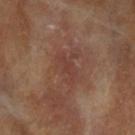Recorded during total-body skin imaging; not selected for excision or biopsy. The lesion is on the left forearm. A region of skin cropped from a whole-body photographic capture, roughly 15 mm wide. A female patient, in their mid-70s.